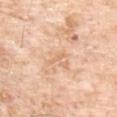Captured during whole-body skin photography for melanoma surveillance; the lesion was not biopsied. This is a white-light tile. A male subject, aged around 70. From the chest. About 2.5 mm across. A 15 mm close-up tile from a total-body photography series done for melanoma screening.